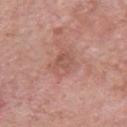The lesion was tiled from a total-body skin photograph and was not biopsied. A lesion tile, about 15 mm wide, cut from a 3D total-body photograph. The recorded lesion diameter is about 3.5 mm. From the chest. A male subject, roughly 55 years of age.